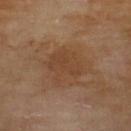{"image": {"source": "total-body photography crop", "field_of_view_mm": 15}, "lighting": "cross-polarized", "site": "upper back", "lesion_size": {"long_diameter_mm_approx": 4.0}, "patient": {"sex": "male", "age_approx": 70}}